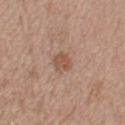Impression:
Part of a total-body skin-imaging series; this lesion was reviewed on a skin check and was not flagged for biopsy.
Context:
The lesion is located on the left upper arm. This is a white-light tile. The recorded lesion diameter is about 2.5 mm. A roughly 15 mm field-of-view crop from a total-body skin photograph. A male patient about 50 years old. The lesion-visualizer software estimated a lesion area of about 4.5 mm², a shape eccentricity near 0.55, and two-axis asymmetry of about 0.15. The software also gave a lesion color around L≈53 a*≈19 b*≈29 in CIELAB, roughly 9 lightness units darker than nearby skin, and a normalized border contrast of about 6.5. The analysis additionally found a border-irregularity rating of about 1.5/10, a color-variation rating of about 2/10, and a peripheral color-asymmetry measure near 1. It also reported an automated nevus-likeness rating near 30 out of 100 and a detector confidence of about 100 out of 100 that the crop contains a lesion.